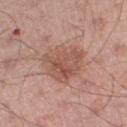Imaged during a routine full-body skin examination; the lesion was not biopsied and no histopathology is available. Captured under white-light illumination. This image is a 15 mm lesion crop taken from a total-body photograph. A male patient approximately 55 years of age. From the right thigh. Measured at roughly 5 mm in maximum diameter.